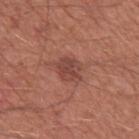No biopsy was performed on this lesion — it was imaged during a full skin examination and was not determined to be concerning.